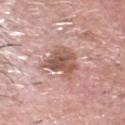Clinical impression: Captured during whole-body skin photography for melanoma surveillance; the lesion was not biopsied. Background: The lesion's longest dimension is about 4.5 mm. A 15 mm close-up tile from a total-body photography series done for melanoma screening. A male subject aged around 70. Located on the head or neck. The total-body-photography lesion software estimated a border-irregularity rating of about 5/10 and a within-lesion color-variation index near 5/10.